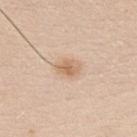{"biopsy_status": "not biopsied; imaged during a skin examination"}